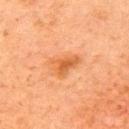<record>
<lesion_size>
  <long_diameter_mm_approx>3.5</long_diameter_mm_approx>
</lesion_size>
<site>right upper arm</site>
<image>
  <source>total-body photography crop</source>
  <field_of_view_mm>15</field_of_view_mm>
</image>
<lighting>cross-polarized</lighting>
<automated_metrics>
  <area_mm2_approx>7.0</area_mm2_approx>
  <eccentricity>0.7</eccentricity>
  <vs_skin_contrast_norm>7.0</vs_skin_contrast_norm>
  <border_irregularity_0_10>4.5</border_irregularity_0_10>
  <color_variation_0_10>2.5</color_variation_0_10>
  <peripheral_color_asymmetry>0.5</peripheral_color_asymmetry>
</automated_metrics>
<patient>
  <sex>female</sex>
  <age_approx>60</age_approx>
</patient>
</record>